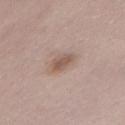Image and clinical context:
A close-up tile cropped from a whole-body skin photograph, about 15 mm across. From the abdomen. A female patient in their 30s. The lesion's longest dimension is about 4 mm. The total-body-photography lesion software estimated an area of roughly 5 mm² and an outline eccentricity of about 0.9 (0 = round, 1 = elongated). The software also gave border irregularity of about 3 on a 0–10 scale, a within-lesion color-variation index near 2/10, and a peripheral color-asymmetry measure near 0.5.